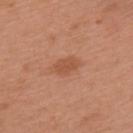No biopsy was performed on this lesion — it was imaged during a full skin examination and was not determined to be concerning. On the upper back. The subject is a female aged 38–42. Captured under white-light illumination. Approximately 2.5 mm at its widest. A 15 mm close-up tile from a total-body photography series done for melanoma screening.